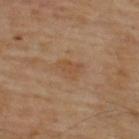biopsy_status: not biopsied; imaged during a skin examination
image:
  source: total-body photography crop
  field_of_view_mm: 15
lesion_size:
  long_diameter_mm_approx: 3.5
patient:
  sex: male
  age_approx: 65
site: back